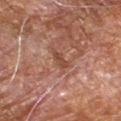| field | value |
|---|---|
| biopsy status | total-body-photography surveillance lesion; no biopsy |
| patient | male, aged 68–72 |
| location | the right forearm |
| acquisition | total-body-photography crop, ~15 mm field of view |
| diameter | ~3 mm (longest diameter) |
| tile lighting | cross-polarized |